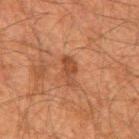The lesion was tiled from a total-body skin photograph and was not biopsied. From the upper back. This image is a 15 mm lesion crop taken from a total-body photograph. Approximately 3.5 mm at its widest. A male subject approximately 60 years of age.